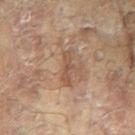Assessment: The lesion was photographed on a routine skin check and not biopsied; there is no pathology result. Context: The lesion is on the arm. A female subject aged 78 to 82. Captured under cross-polarized illumination. A lesion tile, about 15 mm wide, cut from a 3D total-body photograph. The lesion's longest dimension is about 3.5 mm.